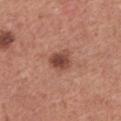Impression:
The lesion was photographed on a routine skin check and not biopsied; there is no pathology result.
Clinical summary:
The lesion's longest dimension is about 3 mm. The subject is a female roughly 35 years of age. The lesion is located on the back. The total-body-photography lesion software estimated a lesion area of about 6 mm² and two-axis asymmetry of about 0.25. The analysis additionally found a border-irregularity rating of about 2.5/10, internal color variation of about 4 on a 0–10 scale, and a peripheral color-asymmetry measure near 1.5. The analysis additionally found an automated nevus-likeness rating near 80 out of 100. A roughly 15 mm field-of-view crop from a total-body skin photograph. The tile uses white-light illumination.